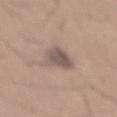Findings:
• notes — catalogued during a skin exam; not biopsied
• imaging modality — 15 mm crop, total-body photography
• illumination — white-light illumination
• location — the mid back
• subject — male, approximately 65 years of age
• TBP lesion metrics — an average lesion color of about L≈53 a*≈14 b*≈20 (CIELAB), a lesion–skin lightness drop of about 11, and a normalized lesion–skin contrast near 8.5; an automated nevus-likeness rating near 15 out of 100 and a lesion-detection confidence of about 100/100
• size — about 4 mm